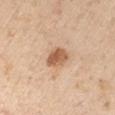Part of a total-body skin-imaging series; this lesion was reviewed on a skin check and was not flagged for biopsy. A male patient aged approximately 75. The lesion-visualizer software estimated an average lesion color of about L≈59 a*≈22 b*≈34 (CIELAB), roughly 14 lightness units darker than nearby skin, and a lesion-to-skin contrast of about 9 (normalized; higher = more distinct). The analysis additionally found an automated nevus-likeness rating near 95 out of 100 and lesion-presence confidence of about 100/100. The lesion is on the arm. Cropped from a total-body skin-imaging series; the visible field is about 15 mm.